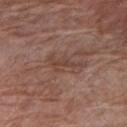Q: Is there a histopathology result?
A: no biopsy performed (imaged during a skin exam)
Q: Patient demographics?
A: female, aged 68–72
Q: What lighting was used for the tile?
A: white-light
Q: How large is the lesion?
A: about 4.5 mm
Q: How was this image acquired?
A: ~15 mm crop, total-body skin-cancer survey
Q: Lesion location?
A: the right upper arm
Q: Automated lesion metrics?
A: a mean CIELAB color near L≈43 a*≈19 b*≈25 and a normalized border contrast of about 6